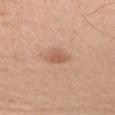Assessment:
Recorded during total-body skin imaging; not selected for excision or biopsy.
Background:
Located on the right upper arm. A male subject roughly 55 years of age. Longest diameter approximately 2.5 mm. A 15 mm close-up tile from a total-body photography series done for melanoma screening. Imaged with white-light lighting.